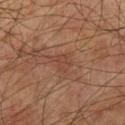Clinical summary:
A male patient, about 75 years old. The tile uses cross-polarized illumination. A region of skin cropped from a whole-body photographic capture, roughly 15 mm wide. From the left upper arm. The recorded lesion diameter is about 2.5 mm.